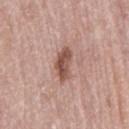{
  "biopsy_status": "not biopsied; imaged during a skin examination",
  "image": {
    "source": "total-body photography crop",
    "field_of_view_mm": 15
  },
  "patient": {
    "sex": "female",
    "age_approx": 60
  },
  "lighting": "white-light",
  "site": "lower back",
  "lesion_size": {
    "long_diameter_mm_approx": 4.0
  }
}